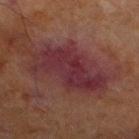Recorded during total-body skin imaging; not selected for excision or biopsy.
Captured under cross-polarized illumination.
A male subject, aged approximately 70.
Longest diameter approximately 7.5 mm.
The lesion is located on the left lower leg.
The lesion-visualizer software estimated an area of roughly 23 mm², an eccentricity of roughly 0.85, and a symmetry-axis asymmetry near 0.4. The software also gave a mean CIELAB color near L≈24 a*≈22 b*≈14 and a normalized border contrast of about 10.5.
A region of skin cropped from a whole-body photographic capture, roughly 15 mm wide.